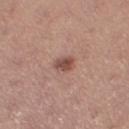Impression: The lesion was tiled from a total-body skin photograph and was not biopsied. Background: A close-up tile cropped from a whole-body skin photograph, about 15 mm across. This is a white-light tile. Approximately 2.5 mm at its widest. On the right thigh. A female subject, roughly 25 years of age. Automated image analysis of the tile measured an eccentricity of roughly 0.65 and a symmetry-axis asymmetry near 0.2. It also reported a lesion color around L≈50 a*≈21 b*≈24 in CIELAB, roughly 11 lightness units darker than nearby skin, and a lesion-to-skin contrast of about 8 (normalized; higher = more distinct). It also reported a lesion-detection confidence of about 100/100.